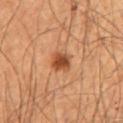Recorded during total-body skin imaging; not selected for excision or biopsy. Located on the chest. The total-body-photography lesion software estimated a footprint of about 5 mm² and an outline eccentricity of about 0.45 (0 = round, 1 = elongated). The analysis additionally found a normalized lesion–skin contrast near 9.5. And it measured border irregularity of about 1.5 on a 0–10 scale, internal color variation of about 3.5 on a 0–10 scale, and a peripheral color-asymmetry measure near 1. The software also gave an automated nevus-likeness rating near 95 out of 100. The tile uses cross-polarized illumination. A male subject, approximately 55 years of age. About 2.5 mm across. A roughly 15 mm field-of-view crop from a total-body skin photograph.